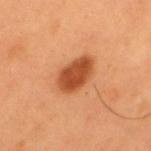The total-body-photography lesion software estimated a shape eccentricity near 0.8 and two-axis asymmetry of about 0.15.
A male patient aged around 55.
The lesion's longest dimension is about 4.5 mm.
A close-up tile cropped from a whole-body skin photograph, about 15 mm across.
From the upper back.